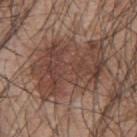Assessment: This lesion was catalogued during total-body skin photography and was not selected for biopsy. Background: On the upper back. Captured under white-light illumination. A male subject roughly 45 years of age. Cropped from a total-body skin-imaging series; the visible field is about 15 mm.